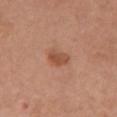Impression:
Part of a total-body skin-imaging series; this lesion was reviewed on a skin check and was not flagged for biopsy.
Context:
A close-up tile cropped from a whole-body skin photograph, about 15 mm across. The subject is a female aged 63 to 67. Automated tile analysis of the lesion measured a lesion area of about 4.5 mm², an eccentricity of roughly 0.7, and a symmetry-axis asymmetry near 0.2. It also reported border irregularity of about 2 on a 0–10 scale, a color-variation rating of about 2.5/10, and peripheral color asymmetry of about 0.5. Approximately 3 mm at its widest. Located on the chest. This is a white-light tile.